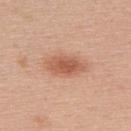Findings:
- lighting · white-light
- patient · female, aged approximately 40
- image-analysis metrics · an average lesion color of about L≈59 a*≈24 b*≈33 (CIELAB), roughly 11 lightness units darker than nearby skin, and a lesion-to-skin contrast of about 7.5 (normalized; higher = more distinct)
- site · the upper back
- image source · 15 mm crop, total-body photography